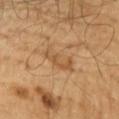acquisition: ~15 mm crop, total-body skin-cancer survey | anatomic site: the chest | patient: male, aged approximately 60 | lesion diameter: about 4.5 mm | illumination: cross-polarized | automated lesion analysis: a lesion area of about 8 mm², an eccentricity of roughly 0.9, and a shape-asymmetry score of about 0.35 (0 = symmetric); internal color variation of about 3.5 on a 0–10 scale and peripheral color asymmetry of about 1; a nevus-likeness score of about 0/100 and lesion-presence confidence of about 100/100.